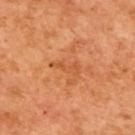| feature | finding |
|---|---|
| image-analysis metrics | a border-irregularity index near 6.5/10, a within-lesion color-variation index near 0/10, and peripheral color asymmetry of about 0; a classifier nevus-likeness of about 0/100 and a lesion-detection confidence of about 100/100 |
| size | ~3.5 mm (longest diameter) |
| patient | male, aged 63 to 67 |
| acquisition | ~15 mm tile from a whole-body skin photo |
| illumination | cross-polarized illumination |